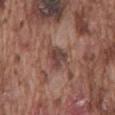The lesion was photographed on a routine skin check and not biopsied; there is no pathology result. Located on the mid back. This image is a 15 mm lesion crop taken from a total-body photograph. A male subject, in their mid-70s. The total-body-photography lesion software estimated a mean CIELAB color near L≈42 a*≈19 b*≈22, about 10 CIELAB-L* units darker than the surrounding skin, and a lesion-to-skin contrast of about 8 (normalized; higher = more distinct). The software also gave a border-irregularity rating of about 3.5/10 and peripheral color asymmetry of about 1. Imaged with white-light lighting.